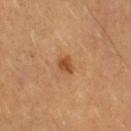biopsy status: no biopsy performed (imaged during a skin exam)
patient: male, in their mid- to late 60s
location: the right upper arm
imaging modality: ~15 mm crop, total-body skin-cancer survey
size: ~2 mm (longest diameter)
TBP lesion metrics: an average lesion color of about L≈44 a*≈22 b*≈36 (CIELAB), a lesion–skin lightness drop of about 10, and a normalized lesion–skin contrast near 8.5; border irregularity of about 2 on a 0–10 scale, internal color variation of about 2.5 on a 0–10 scale, and a peripheral color-asymmetry measure near 1; lesion-presence confidence of about 100/100
lighting: cross-polarized